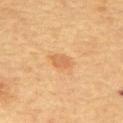Captured during whole-body skin photography for melanoma surveillance; the lesion was not biopsied. Measured at roughly 3 mm in maximum diameter. On the back. Imaged with cross-polarized lighting. The lesion-visualizer software estimated a within-lesion color-variation index near 2.5/10. A 15 mm close-up tile from a total-body photography series done for melanoma screening. A female patient roughly 60 years of age.